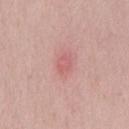follow-up: no biopsy performed (imaged during a skin exam) | subject: male, aged approximately 40 | imaging modality: ~15 mm tile from a whole-body skin photo | location: the chest | diameter: ~2.5 mm (longest diameter).